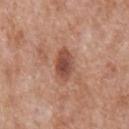biopsy status: no biopsy performed (imaged during a skin exam); subject: male, about 65 years old; image: 15 mm crop, total-body photography; site: the abdomen; image-analysis metrics: a lesion area of about 7.5 mm² and an outline eccentricity of about 0.8 (0 = round, 1 = elongated); lighting: white-light; size: ≈4 mm.